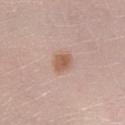notes — imaged on a skin check; not biopsied
diameter — ≈2.5 mm
image-analysis metrics — a lesion color around L≈58 a*≈20 b*≈29 in CIELAB and about 10 CIELAB-L* units darker than the surrounding skin; internal color variation of about 2.5 on a 0–10 scale and a peripheral color-asymmetry measure near 0.5; a nevus-likeness score of about 95/100
patient — female, roughly 35 years of age
anatomic site — the left lower leg
lighting — white-light
image source — total-body-photography crop, ~15 mm field of view